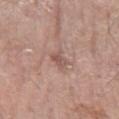workup = catalogued during a skin exam; not biopsied
tile lighting = white-light illumination
patient = male, about 60 years old
lesion diameter = about 2.5 mm
anatomic site = the left forearm
image = ~15 mm tile from a whole-body skin photo